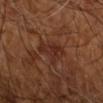biopsy status — catalogued during a skin exam; not biopsied | tile lighting — cross-polarized | body site — the right upper arm | patient — in their mid- to late 60s | image source — 15 mm crop, total-body photography | size — about 4 mm | automated metrics — a lesion area of about 7 mm² and an outline eccentricity of about 0.75 (0 = round, 1 = elongated); a lesion color around L≈28 a*≈22 b*≈26 in CIELAB and about 6 CIELAB-L* units darker than the surrounding skin; a color-variation rating of about 3/10 and peripheral color asymmetry of about 1; a classifier nevus-likeness of about 0/100 and lesion-presence confidence of about 95/100.